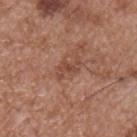  biopsy_status: not biopsied; imaged during a skin examination
  image:
    source: total-body photography crop
    field_of_view_mm: 15
  site: chest
  patient:
    sex: male
    age_approx: 55